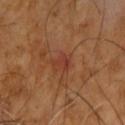Recorded during total-body skin imaging; not selected for excision or biopsy.